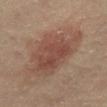Notes:
– biopsy status: total-body-photography surveillance lesion; no biopsy
– site: the abdomen
– TBP lesion metrics: a lesion color around L≈45 a*≈19 b*≈25 in CIELAB, roughly 8 lightness units darker than nearby skin, and a normalized border contrast of about 6.5; a border-irregularity index near 4.5/10, a within-lesion color-variation index near 4/10, and peripheral color asymmetry of about 1; a classifier nevus-likeness of about 90/100 and a lesion-detection confidence of about 100/100
– size: ~7.5 mm (longest diameter)
– patient: male, approximately 85 years of age or older
– image source: 15 mm crop, total-body photography
– lighting: cross-polarized illumination